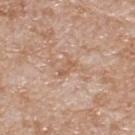No biopsy was performed on this lesion — it was imaged during a full skin examination and was not determined to be concerning.